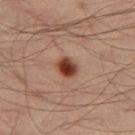Assessment:
Captured during whole-body skin photography for melanoma surveillance; the lesion was not biopsied.
Image and clinical context:
A region of skin cropped from a whole-body photographic capture, roughly 15 mm wide. On the leg. The lesion's longest dimension is about 2.5 mm. A male patient, approximately 35 years of age. Automated tile analysis of the lesion measured an outline eccentricity of about 0.6 (0 = round, 1 = elongated) and a shape-asymmetry score of about 0.2 (0 = symmetric). It also reported border irregularity of about 1.5 on a 0–10 scale, a within-lesion color-variation index near 6/10, and peripheral color asymmetry of about 2. The software also gave an automated nevus-likeness rating near 100 out of 100.